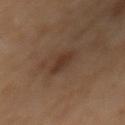biopsy_status: not biopsied; imaged during a skin examination
patient:
  sex: female
  age_approx: 50
lighting: cross-polarized
automated_metrics:
  cielab_L: 33
  cielab_a: 17
  cielab_b: 26
  vs_skin_darker_L: 8.0
  vs_skin_contrast_norm: 7.5
  nevus_likeness_0_100: 70
  lesion_detection_confidence_0_100: 100
site: mid back
image:
  source: total-body photography crop
  field_of_view_mm: 15
lesion_size:
  long_diameter_mm_approx: 4.5